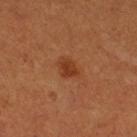Q: Is there a histopathology result?
A: no biopsy performed (imaged during a skin exam)
Q: What is the lesion's diameter?
A: ~2.5 mm (longest diameter)
Q: What is the anatomic site?
A: the right thigh
Q: Who is the patient?
A: male, aged around 50
Q: What kind of image is this?
A: ~15 mm crop, total-body skin-cancer survey